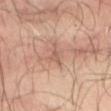Part of a total-body skin-imaging series; this lesion was reviewed on a skin check and was not flagged for biopsy.
Imaged with cross-polarized lighting.
A patient aged around 65.
A 15 mm close-up tile from a total-body photography series done for melanoma screening.
The lesion is on the abdomen.
Automated tile analysis of the lesion measured an area of roughly 3 mm², an eccentricity of roughly 0.85, and two-axis asymmetry of about 0.55. The software also gave a mean CIELAB color near L≈56 a*≈20 b*≈27, roughly 8 lightness units darker than nearby skin, and a normalized border contrast of about 5.
Longest diameter approximately 3 mm.